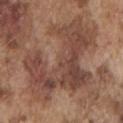notes: imaged on a skin check; not biopsied | automated lesion analysis: an area of roughly 50 mm², an eccentricity of roughly 0.75, and a shape-asymmetry score of about 0.6 (0 = symmetric); a mean CIELAB color near L≈45 a*≈20 b*≈26 and a normalized border contrast of about 7.5 | size: about 10 mm | patient: male, aged 73 to 77 | tile lighting: white-light | anatomic site: the left upper arm | image: total-body-photography crop, ~15 mm field of view.